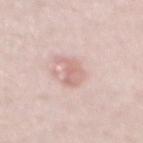Findings:
• image · ~15 mm crop, total-body skin-cancer survey
• site · the chest
• subject · male, aged approximately 40